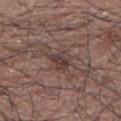The lesion-visualizer software estimated a lesion area of about 5.5 mm², an eccentricity of roughly 0.35, and two-axis asymmetry of about 0.35. And it measured an average lesion color of about L≈39 a*≈16 b*≈19 (CIELAB) and a lesion–skin lightness drop of about 8. And it measured a border-irregularity index near 3.5/10, internal color variation of about 4 on a 0–10 scale, and peripheral color asymmetry of about 1.5. A male patient in their 70s. A 15 mm close-up extracted from a 3D total-body photography capture. The lesion's longest dimension is about 3 mm. Imaged with white-light lighting. On the left arm.